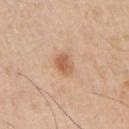<lesion>
  <biopsy_status>not biopsied; imaged during a skin examination</biopsy_status>
  <lighting>white-light</lighting>
  <patient>
    <sex>male</sex>
    <age_approx>65</age_approx>
  </patient>
  <image>
    <source>total-body photography crop</source>
    <field_of_view_mm>15</field_of_view_mm>
  </image>
  <lesion_size>
    <long_diameter_mm_approx>2.5</long_diameter_mm_approx>
  </lesion_size>
  <site>left upper arm</site>
</lesion>